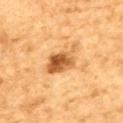Clinical impression:
Imaged during a routine full-body skin examination; the lesion was not biopsied and no histopathology is available.
Image and clinical context:
Imaged with cross-polarized lighting. Longest diameter approximately 4.5 mm. A male subject approximately 85 years of age or older. A roughly 15 mm field-of-view crop from a total-body skin photograph. Automated tile analysis of the lesion measured an area of roughly 8.5 mm², a shape eccentricity near 0.8, and two-axis asymmetry of about 0.25. It also reported an automated nevus-likeness rating near 85 out of 100 and a lesion-detection confidence of about 100/100. On the upper back.